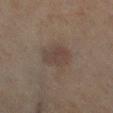The lesion was photographed on a routine skin check and not biopsied; there is no pathology result. A female subject aged approximately 60. A 15 mm crop from a total-body photograph taken for skin-cancer surveillance. Captured under cross-polarized illumination. The lesion is on the right lower leg. Longest diameter approximately 4 mm.